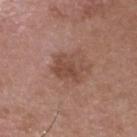Q: Was a biopsy performed?
A: total-body-photography surveillance lesion; no biopsy
Q: Where on the body is the lesion?
A: the head or neck
Q: Lesion size?
A: about 4 mm
Q: What is the imaging modality?
A: ~15 mm crop, total-body skin-cancer survey
Q: Patient demographics?
A: male, about 50 years old